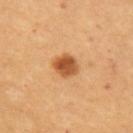Impression: Imaged during a routine full-body skin examination; the lesion was not biopsied and no histopathology is available. Image and clinical context: On the left upper arm. A female patient, roughly 45 years of age. Automated tile analysis of the lesion measured an outline eccentricity of about 0.6 (0 = round, 1 = elongated) and a symmetry-axis asymmetry near 0.2. And it measured an automated nevus-likeness rating near 100 out of 100 and lesion-presence confidence of about 100/100. About 3 mm across. Imaged with cross-polarized lighting. This image is a 15 mm lesion crop taken from a total-body photograph.